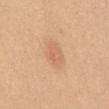Assessment:
The lesion was tiled from a total-body skin photograph and was not biopsied.
Image and clinical context:
A 15 mm close-up extracted from a 3D total-body photography capture. A male subject about 50 years old. On the front of the torso.